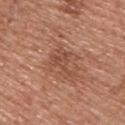Q: Was a biopsy performed?
A: total-body-photography surveillance lesion; no biopsy
Q: Where on the body is the lesion?
A: the upper back
Q: Automated lesion metrics?
A: a shape-asymmetry score of about 0.6 (0 = symmetric); a lesion color around L≈49 a*≈23 b*≈31 in CIELAB and roughly 8 lightness units darker than nearby skin
Q: Patient demographics?
A: male, roughly 55 years of age
Q: What kind of image is this?
A: total-body-photography crop, ~15 mm field of view